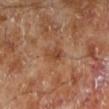Q: Was this lesion biopsied?
A: no biopsy performed (imaged during a skin exam)
Q: Automated lesion metrics?
A: a footprint of about 4 mm² and a symmetry-axis asymmetry near 0.3; a mean CIELAB color near L≈43 a*≈22 b*≈32 and roughly 7 lightness units darker than nearby skin; an automated nevus-likeness rating near 0 out of 100 and lesion-presence confidence of about 100/100
Q: What is the anatomic site?
A: the left lower leg
Q: What lighting was used for the tile?
A: cross-polarized
Q: How was this image acquired?
A: 15 mm crop, total-body photography
Q: Who is the patient?
A: male, about 70 years old